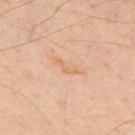<lesion>
<biopsy_status>not biopsied; imaged during a skin examination</biopsy_status>
<automated_metrics>
  <border_irregularity_0_10>5.0</border_irregularity_0_10>
  <peripheral_color_asymmetry>0.0</peripheral_color_asymmetry>
  <nevus_likeness_0_100>0</nevus_likeness_0_100>
  <lesion_detection_confidence_0_100>100</lesion_detection_confidence_0_100>
</automated_metrics>
<lesion_size>
  <long_diameter_mm_approx>4.0</long_diameter_mm_approx>
</lesion_size>
<site>mid back</site>
<image>
  <source>total-body photography crop</source>
  <field_of_view_mm>15</field_of_view_mm>
</image>
<patient>
  <sex>male</sex>
  <age_approx>45</age_approx>
</patient>
<lighting>cross-polarized</lighting>
</lesion>